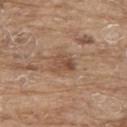Case summary:
– follow-up: no biopsy performed (imaged during a skin exam)
– image: ~15 mm crop, total-body skin-cancer survey
– location: the back
– patient: male, in their 80s
– automated metrics: a lesion area of about 6.5 mm², an eccentricity of roughly 0.55, and a shape-asymmetry score of about 0.3 (0 = symmetric); a lesion color around L≈50 a*≈18 b*≈30 in CIELAB and a lesion–skin lightness drop of about 9; a border-irregularity rating of about 2.5/10, a within-lesion color-variation index near 5/10, and a peripheral color-asymmetry measure near 2; a lesion-detection confidence of about 100/100